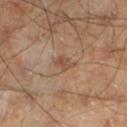Q: Is there a histopathology result?
A: imaged on a skin check; not biopsied
Q: How was this image acquired?
A: 15 mm crop, total-body photography
Q: Lesion location?
A: the left lower leg
Q: Who is the patient?
A: male, roughly 45 years of age
Q: Illumination type?
A: cross-polarized illumination